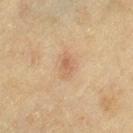{
  "biopsy_status": "not biopsied; imaged during a skin examination",
  "lighting": "cross-polarized",
  "site": "left thigh",
  "lesion_size": {
    "long_diameter_mm_approx": 3.0
  },
  "image": {
    "source": "total-body photography crop",
    "field_of_view_mm": 15
  },
  "patient": {
    "sex": "female",
    "age_approx": 55
  }
}